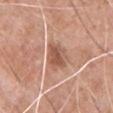This lesion was catalogued during total-body skin photography and was not selected for biopsy. Imaged with white-light lighting. The recorded lesion diameter is about 4 mm. The lesion is located on the chest. A male patient, approximately 60 years of age. A roughly 15 mm field-of-view crop from a total-body skin photograph.